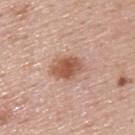The lesion was photographed on a routine skin check and not biopsied; there is no pathology result.
This image is a 15 mm lesion crop taken from a total-body photograph.
The subject is a male in their mid-50s.
Located on the upper back.